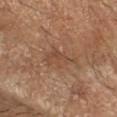Clinical impression:
The lesion was tiled from a total-body skin photograph and was not biopsied.
Image and clinical context:
Cropped from a whole-body photographic skin survey; the tile spans about 15 mm. An algorithmic analysis of the crop reported a lesion area of about 6.5 mm² and a symmetry-axis asymmetry near 0.4. The analysis additionally found a color-variation rating of about 2/10 and radial color variation of about 1. The lesion is on the arm. A male subject, about 65 years old. Measured at roughly 4.5 mm in maximum diameter. This is a cross-polarized tile.